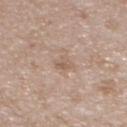This lesion was catalogued during total-body skin photography and was not selected for biopsy.
Imaged with white-light lighting.
The lesion is located on the upper back.
A female patient, approximately 30 years of age.
A region of skin cropped from a whole-body photographic capture, roughly 15 mm wide.